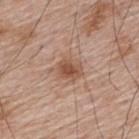| key | value |
|---|---|
| follow-up | no biopsy performed (imaged during a skin exam) |
| patient | male, aged 63–67 |
| imaging modality | 15 mm crop, total-body photography |
| body site | the back |
| illumination | white-light |